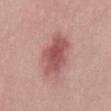patient: male, aged approximately 30; image source: ~15 mm tile from a whole-body skin photo.